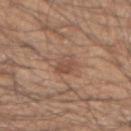<case>
  <image>
    <source>total-body photography crop</source>
    <field_of_view_mm>15</field_of_view_mm>
  </image>
  <automated_metrics>
    <lesion_detection_confidence_0_100>100</lesion_detection_confidence_0_100>
  </automated_metrics>
  <lesion_size>
    <long_diameter_mm_approx>3.0</long_diameter_mm_approx>
  </lesion_size>
  <site>right forearm</site>
  <patient>
    <sex>male</sex>
    <age_approx>50</age_approx>
  </patient>
</case>